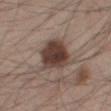Case summary:
– notes: no biopsy performed (imaged during a skin exam)
– image source: total-body-photography crop, ~15 mm field of view
– subject: male, approximately 45 years of age
– lighting: white-light illumination
– TBP lesion metrics: a footprint of about 14 mm², an eccentricity of roughly 0.35, and a shape-asymmetry score of about 0.2 (0 = symmetric); a mean CIELAB color near L≈40 a*≈16 b*≈22, about 15 CIELAB-L* units darker than the surrounding skin, and a normalized lesion–skin contrast near 12; a classifier nevus-likeness of about 90/100 and a detector confidence of about 100 out of 100 that the crop contains a lesion
– anatomic site: the right thigh
– lesion diameter: ~4.5 mm (longest diameter)